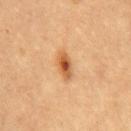Q: Was a biopsy performed?
A: total-body-photography surveillance lesion; no biopsy
Q: What is the anatomic site?
A: the chest
Q: What lighting was used for the tile?
A: cross-polarized illumination
Q: Lesion size?
A: about 3.5 mm
Q: Patient demographics?
A: female, aged around 55
Q: How was this image acquired?
A: ~15 mm crop, total-body skin-cancer survey